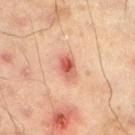Impression:
This lesion was catalogued during total-body skin photography and was not selected for biopsy.
Background:
Captured under cross-polarized illumination. About 3 mm across. A male patient in their mid- to late 40s. On the left lower leg. A 15 mm crop from a total-body photograph taken for skin-cancer surveillance.